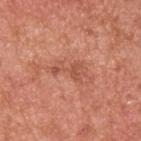Cropped from a whole-body photographic skin survey; the tile spans about 15 mm. Automated image analysis of the tile measured a footprint of about 5 mm² and a shape-asymmetry score of about 0.6 (0 = symmetric). And it measured a lesion color around L≈53 a*≈28 b*≈32 in CIELAB and about 8 CIELAB-L* units darker than the surrounding skin. It also reported a border-irregularity index near 8/10, a within-lesion color-variation index near 2/10, and radial color variation of about 0.5. The lesion is located on the upper back. Measured at roughly 4 mm in maximum diameter. Imaged with white-light lighting. A male patient, approximately 55 years of age.